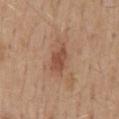Q: Was a biopsy performed?
A: no biopsy performed (imaged during a skin exam)
Q: How large is the lesion?
A: ≈4 mm
Q: What is the imaging modality?
A: total-body-photography crop, ~15 mm field of view
Q: What are the patient's age and sex?
A: male, approximately 55 years of age
Q: Where on the body is the lesion?
A: the mid back